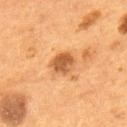workup: total-body-photography surveillance lesion; no biopsy | size: ~3.5 mm (longest diameter) | automated lesion analysis: an area of roughly 7.5 mm², an outline eccentricity of about 0.55 (0 = round, 1 = elongated), and two-axis asymmetry of about 0.2; a lesion color around L≈54 a*≈24 b*≈40 in CIELAB, roughly 12 lightness units darker than nearby skin, and a lesion-to-skin contrast of about 8.5 (normalized; higher = more distinct); a border-irregularity index near 1.5/10 and peripheral color asymmetry of about 1.5; a classifier nevus-likeness of about 80/100 and a detector confidence of about 100 out of 100 that the crop contains a lesion | lighting: cross-polarized | acquisition: 15 mm crop, total-body photography | subject: male, approximately 55 years of age | location: the upper back.